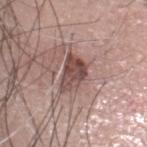Assessment:
The lesion was photographed on a routine skin check and not biopsied; there is no pathology result.
Acquisition and patient details:
Cropped from a whole-body photographic skin survey; the tile spans about 15 mm. The patient is a male about 60 years old. The lesion is located on the head or neck. The total-body-photography lesion software estimated a nevus-likeness score of about 20/100.